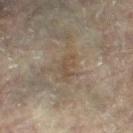<case>
<biopsy_status>not biopsied; imaged during a skin examination</biopsy_status>
<lighting>cross-polarized</lighting>
<patient>
  <sex>female</sex>
  <age_approx>80</age_approx>
</patient>
<image>
  <source>total-body photography crop</source>
  <field_of_view_mm>15</field_of_view_mm>
</image>
<site>right leg</site>
<lesion_size>
  <long_diameter_mm_approx>3.0</long_diameter_mm_approx>
</lesion_size>
</case>